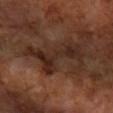Clinical impression: The lesion was photographed on a routine skin check and not biopsied; there is no pathology result. Image and clinical context: Captured under cross-polarized illumination. The lesion is located on the arm. A 15 mm crop from a total-body photograph taken for skin-cancer surveillance. A female subject in their mid- to late 60s. Approximately 6.5 mm at its widest.